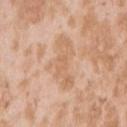Part of a total-body skin-imaging series; this lesion was reviewed on a skin check and was not flagged for biopsy.
The lesion is located on the arm.
Cropped from a whole-body photographic skin survey; the tile spans about 15 mm.
A female subject, aged 23–27.